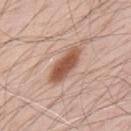Assessment:
The lesion was photographed on a routine skin check and not biopsied; there is no pathology result.
Clinical summary:
On the upper back. The patient is a male aged approximately 70. The tile uses white-light illumination. This image is a 15 mm lesion crop taken from a total-body photograph. Automated image analysis of the tile measured a color-variation rating of about 4/10 and radial color variation of about 1. It also reported a classifier nevus-likeness of about 95/100 and lesion-presence confidence of about 100/100.